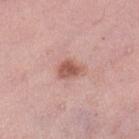{"biopsy_status": "not biopsied; imaged during a skin examination"}